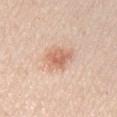Case summary:
* follow-up — catalogued during a skin exam; not biopsied
* image — 15 mm crop, total-body photography
* anatomic site — the right upper arm
* subject — female, in their mid-50s
* lesion size — ≈4 mm
* automated lesion analysis — an area of roughly 8 mm² and a symmetry-axis asymmetry near 0.25; a within-lesion color-variation index near 4/10 and peripheral color asymmetry of about 1